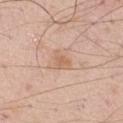Imaged with white-light lighting.
About 3 mm across.
A male subject aged 58 to 62.
On the right thigh.
A close-up tile cropped from a whole-body skin photograph, about 15 mm across.